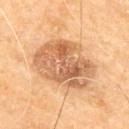Q: Is there a histopathology result?
A: imaged on a skin check; not biopsied
Q: What kind of image is this?
A: ~15 mm tile from a whole-body skin photo
Q: What is the anatomic site?
A: the arm
Q: Patient demographics?
A: male, aged around 70
Q: What lighting was used for the tile?
A: cross-polarized